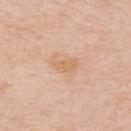Case summary:
- follow-up — total-body-photography surveillance lesion; no biopsy
- lesion diameter — ≈3 mm
- patient — male, aged around 75
- body site — the upper back
- lighting — white-light illumination
- image-analysis metrics — an outline eccentricity of about 0.8 (0 = round, 1 = elongated) and a shape-asymmetry score of about 0.35 (0 = symmetric); a nevus-likeness score of about 0/100
- image — ~15 mm tile from a whole-body skin photo